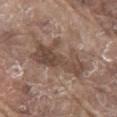Assessment: Part of a total-body skin-imaging series; this lesion was reviewed on a skin check and was not flagged for biopsy. Acquisition and patient details: A male patient aged approximately 80. Located on the abdomen. Captured under white-light illumination. A close-up tile cropped from a whole-body skin photograph, about 15 mm across. Automated tile analysis of the lesion measured a footprint of about 20 mm², an eccentricity of roughly 0.9, and a symmetry-axis asymmetry near 0.45. The analysis additionally found a border-irregularity rating of about 7.5/10, internal color variation of about 4.5 on a 0–10 scale, and peripheral color asymmetry of about 1.5. The software also gave an automated nevus-likeness rating near 0 out of 100 and a lesion-detection confidence of about 65/100.